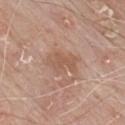  biopsy_status: not biopsied; imaged during a skin examination
  automated_metrics:
    area_mm2_approx: 7.0
    eccentricity: 0.8
    shape_asymmetry: 0.4
    cielab_L: 55
    cielab_a: 20
    cielab_b: 29
    vs_skin_contrast_norm: 5.5
  image:
    source: total-body photography crop
    field_of_view_mm: 15
  site: front of the torso
  patient:
    sex: male
    age_approx: 80